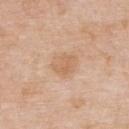follow-up = total-body-photography surveillance lesion; no biopsy | lesion size = ~3.5 mm (longest diameter) | subject = male, approximately 75 years of age | automated metrics = a border-irregularity rating of about 2.5/10, a color-variation rating of about 2.5/10, and radial color variation of about 1; an automated nevus-likeness rating near 0 out of 100 | image = 15 mm crop, total-body photography | tile lighting = white-light | anatomic site = the upper back.